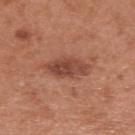Impression:
This lesion was catalogued during total-body skin photography and was not selected for biopsy.
Clinical summary:
Captured under white-light illumination. Located on the upper back. A male subject aged approximately 55. A region of skin cropped from a whole-body photographic capture, roughly 15 mm wide. The lesion's longest dimension is about 5.5 mm. Automated image analysis of the tile measured an area of roughly 9 mm², an outline eccentricity of about 0.9 (0 = round, 1 = elongated), and a shape-asymmetry score of about 0.2 (0 = symmetric). The software also gave a within-lesion color-variation index near 4/10.